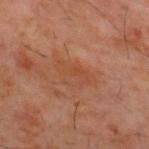The tile uses cross-polarized illumination. A male patient aged 58 to 62. Automated tile analysis of the lesion measured an eccentricity of roughly 0.85 and a symmetry-axis asymmetry near 0.35. The software also gave an average lesion color of about L≈44 a*≈23 b*≈32 (CIELAB), roughly 5 lightness units darker than nearby skin, and a lesion-to-skin contrast of about 5 (normalized; higher = more distinct). The analysis additionally found internal color variation of about 4 on a 0–10 scale and a peripheral color-asymmetry measure near 1.5. On the upper back. About 4.5 mm across. A 15 mm close-up tile from a total-body photography series done for melanoma screening.